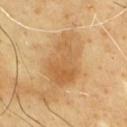The lesion was tiled from a total-body skin photograph and was not biopsied. A male subject, approximately 65 years of age. Captured under cross-polarized illumination. Longest diameter approximately 6.5 mm. The lesion is located on the front of the torso. A 15 mm close-up extracted from a 3D total-body photography capture.